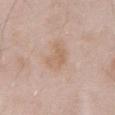Captured during whole-body skin photography for melanoma surveillance; the lesion was not biopsied.
A close-up tile cropped from a whole-body skin photograph, about 15 mm across.
From the abdomen.
The patient is a male in their mid- to late 50s.